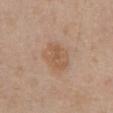workup=catalogued during a skin exam; not biopsied.